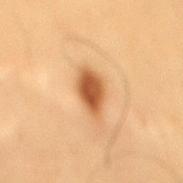Notes:
• workup: no biopsy performed (imaged during a skin exam)
• subject: male, in their mid- to late 50s
• image: total-body-photography crop, ~15 mm field of view
• lighting: cross-polarized
• lesion size: about 4.5 mm
• site: the back
• automated metrics: an eccentricity of roughly 0.85 and a symmetry-axis asymmetry near 0.2; a mean CIELAB color near L≈56 a*≈24 b*≈41 and roughly 16 lightness units darker than nearby skin; border irregularity of about 2 on a 0–10 scale, a color-variation rating of about 4/10, and peripheral color asymmetry of about 1; a detector confidence of about 100 out of 100 that the crop contains a lesion